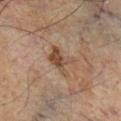Assessment: Part of a total-body skin-imaging series; this lesion was reviewed on a skin check and was not flagged for biopsy. Image and clinical context: This image is a 15 mm lesion crop taken from a total-body photograph. The lesion's longest dimension is about 3.5 mm. A male patient, aged 58–62. From the leg.